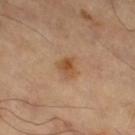<tbp_lesion>
  <biopsy_status>not biopsied; imaged during a skin examination</biopsy_status>
  <lighting>cross-polarized</lighting>
  <lesion_size>
    <long_diameter_mm_approx>2.5</long_diameter_mm_approx>
  </lesion_size>
  <automated_metrics>
    <nevus_likeness_0_100>85</nevus_likeness_0_100>
    <lesion_detection_confidence_0_100>100</lesion_detection_confidence_0_100>
  </automated_metrics>
  <site>right thigh</site>
  <image>
    <source>total-body photography crop</source>
    <field_of_view_mm>15</field_of_view_mm>
  </image>
</tbp_lesion>